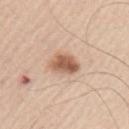Q: Was a biopsy performed?
A: no biopsy performed (imaged during a skin exam)
Q: Where on the body is the lesion?
A: the upper back
Q: What did automated image analysis measure?
A: a border-irregularity index near 2/10, internal color variation of about 5.5 on a 0–10 scale, and peripheral color asymmetry of about 2; an automated nevus-likeness rating near 90 out of 100 and a detector confidence of about 100 out of 100 that the crop contains a lesion
Q: What is the lesion's diameter?
A: ~4 mm (longest diameter)
Q: What are the patient's age and sex?
A: male, approximately 45 years of age
Q: Illumination type?
A: white-light illumination
Q: What is the imaging modality?
A: ~15 mm tile from a whole-body skin photo